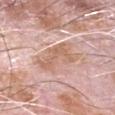Impression:
No biopsy was performed on this lesion — it was imaged during a full skin examination and was not determined to be concerning.
Image and clinical context:
A male patient aged approximately 80. Cropped from a whole-body photographic skin survey; the tile spans about 15 mm. Located on the head or neck.